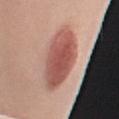Q: Was a biopsy performed?
A: catalogued during a skin exam; not biopsied
Q: Automated lesion metrics?
A: a shape-asymmetry score of about 0.15 (0 = symmetric); border irregularity of about 2 on a 0–10 scale and peripheral color asymmetry of about 1.5
Q: Lesion location?
A: the right upper arm
Q: Patient demographics?
A: female, approximately 50 years of age
Q: What kind of image is this?
A: ~15 mm crop, total-body skin-cancer survey
Q: What lighting was used for the tile?
A: white-light
Q: What is the lesion's diameter?
A: ~6.5 mm (longest diameter)